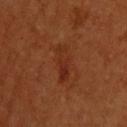<tbp_lesion>
  <biopsy_status>not biopsied; imaged during a skin examination</biopsy_status>
  <image>
    <source>total-body photography crop</source>
    <field_of_view_mm>15</field_of_view_mm>
  </image>
  <lesion_size>
    <long_diameter_mm_approx>4.5</long_diameter_mm_approx>
  </lesion_size>
  <site>upper back</site>
  <patient>
    <sex>male</sex>
    <age_approx>60</age_approx>
  </patient>
  <lighting>cross-polarized</lighting>
  <automated_metrics>
    <area_mm2_approx>6.5</area_mm2_approx>
    <eccentricity>0.9</eccentricity>
    <shape_asymmetry>0.3</shape_asymmetry>
    <color_variation_0_10>2.0</color_variation_0_10>
    <peripheral_color_asymmetry>0.5</peripheral_color_asymmetry>
  </automated_metrics>
</tbp_lesion>